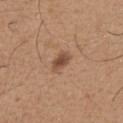  biopsy_status: not biopsied; imaged during a skin examination